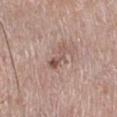Impression: The lesion was tiled from a total-body skin photograph and was not biopsied. Context: A male subject, about 75 years old. Automated tile analysis of the lesion measured an eccentricity of roughly 0.95 and a shape-asymmetry score of about 0.4 (0 = symmetric). And it measured a border-irregularity index near 5/10, internal color variation of about 0.5 on a 0–10 scale, and peripheral color asymmetry of about 0. It also reported a nevus-likeness score of about 20/100 and a detector confidence of about 100 out of 100 that the crop contains a lesion. About 3.5 mm across. This image is a 15 mm lesion crop taken from a total-body photograph. Located on the right lower leg. This is a white-light tile.